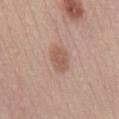A female subject, in their 50s. A 15 mm close-up tile from a total-body photography series done for melanoma screening. The recorded lesion diameter is about 3.5 mm. Located on the mid back. Imaged with white-light lighting.